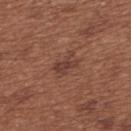Clinical impression: Recorded during total-body skin imaging; not selected for excision or biopsy. Clinical summary: The patient is a female aged approximately 65. From the upper back. Cropped from a whole-body photographic skin survey; the tile spans about 15 mm.